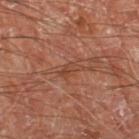– notes · catalogued during a skin exam; not biopsied
– patient · male, aged 68–72
– tile lighting · cross-polarized illumination
– image source · ~15 mm crop, total-body skin-cancer survey
– body site · the left thigh
– diameter · ~3 mm (longest diameter)